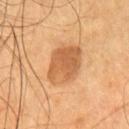<case>
<biopsy_status>not biopsied; imaged during a skin examination</biopsy_status>
<automated_metrics>
  <area_mm2_approx>14.0</area_mm2_approx>
  <eccentricity>0.7</eccentricity>
  <shape_asymmetry>0.2</shape_asymmetry>
  <cielab_L>54</cielab_L>
  <cielab_a>22</cielab_a>
  <cielab_b>38</cielab_b>
  <vs_skin_darker_L>11.0</vs_skin_darker_L>
  <vs_skin_contrast_norm>7.5</vs_skin_contrast_norm>
  <border_irregularity_0_10>2.0</border_irregularity_0_10>
  <color_variation_0_10>4.0</color_variation_0_10>
  <nevus_likeness_0_100>70</nevus_likeness_0_100>
  <lesion_detection_confidence_0_100>100</lesion_detection_confidence_0_100>
</automated_metrics>
<patient>
  <sex>male</sex>
  <age_approx>55</age_approx>
</patient>
<image>
  <source>total-body photography crop</source>
  <field_of_view_mm>15</field_of_view_mm>
</image>
<lesion_size>
  <long_diameter_mm_approx>5.0</long_diameter_mm_approx>
</lesion_size>
<site>chest</site>
<lighting>cross-polarized</lighting>
</case>